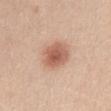Captured during whole-body skin photography for melanoma surveillance; the lesion was not biopsied. Imaged with white-light lighting. A female subject aged around 25. From the chest. An algorithmic analysis of the crop reported a border-irregularity rating of about 1.5/10, a within-lesion color-variation index near 4/10, and peripheral color asymmetry of about 1. A roughly 15 mm field-of-view crop from a total-body skin photograph.